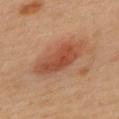Case summary:
• anatomic site · the upper back
• subject · female, approximately 40 years of age
• tile lighting · cross-polarized
• acquisition · ~15 mm crop, total-body skin-cancer survey
• lesion size · about 6.5 mm